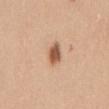Imaged during a routine full-body skin examination; the lesion was not biopsied and no histopathology is available.
From the mid back.
Measured at roughly 3.5 mm in maximum diameter.
A female patient roughly 30 years of age.
Imaged with white-light lighting.
A lesion tile, about 15 mm wide, cut from a 3D total-body photograph.
Automated image analysis of the tile measured a footprint of about 5 mm², an outline eccentricity of about 0.8 (0 = round, 1 = elongated), and a symmetry-axis asymmetry near 0.25. It also reported an average lesion color of about L≈57 a*≈22 b*≈33 (CIELAB) and a normalized border contrast of about 9.5. The analysis additionally found a border-irregularity rating of about 2.5/10 and radial color variation of about 1.